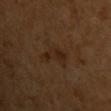follow-up: imaged on a skin check; not biopsied | anatomic site: the arm | automated metrics: a lesion area of about 5.5 mm², an eccentricity of roughly 0.9, and a shape-asymmetry score of about 0.45 (0 = symmetric); a lesion color around L≈24 a*≈16 b*≈26 in CIELAB; a border-irregularity index near 5/10, internal color variation of about 2.5 on a 0–10 scale, and radial color variation of about 1 | illumination: cross-polarized illumination | subject: female, roughly 55 years of age | lesion size: about 4 mm | image source: 15 mm crop, total-body photography.